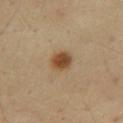Clinical impression:
No biopsy was performed on this lesion — it was imaged during a full skin examination and was not determined to be concerning.
Clinical summary:
Located on the mid back. This is a cross-polarized tile. The subject is a male approximately 55 years of age. Longest diameter approximately 3 mm. A 15 mm close-up tile from a total-body photography series done for melanoma screening. Automated tile analysis of the lesion measured an area of roughly 5.5 mm², an outline eccentricity of about 0.5 (0 = round, 1 = elongated), and a shape-asymmetry score of about 0.15 (0 = symmetric). The software also gave about 13 CIELAB-L* units darker than the surrounding skin. The analysis additionally found a border-irregularity index near 1.5/10, a within-lesion color-variation index near 4/10, and peripheral color asymmetry of about 1.